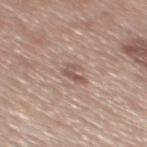biopsy_status: not biopsied; imaged during a skin examination